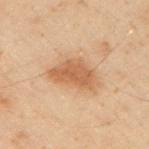A male patient, roughly 50 years of age. Measured at roughly 5.5 mm in maximum diameter. A 15 mm crop from a total-body photograph taken for skin-cancer surveillance. From the left arm. The tile uses cross-polarized illumination.